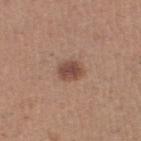Imaged during a routine full-body skin examination; the lesion was not biopsied and no histopathology is available.
About 2.5 mm across.
Located on the left thigh.
Automated tile analysis of the lesion measured a footprint of about 5.5 mm² and a shape-asymmetry score of about 0.15 (0 = symmetric). And it measured a normalized lesion–skin contrast near 8.5. The analysis additionally found an automated nevus-likeness rating near 90 out of 100 and a lesion-detection confidence of about 100/100.
A female subject aged approximately 40.
A region of skin cropped from a whole-body photographic capture, roughly 15 mm wide.
This is a white-light tile.